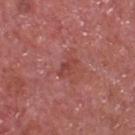notes — imaged on a skin check; not biopsied | subject — male, in their 70s | lesion diameter — ~3 mm (longest diameter) | body site — the front of the torso | imaging modality — ~15 mm tile from a whole-body skin photo | lighting — white-light illumination.